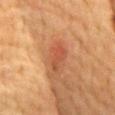Acquisition and patient details: A male subject aged 83 or over. Imaged with cross-polarized lighting. Approximately 3.5 mm at its widest. A lesion tile, about 15 mm wide, cut from a 3D total-body photograph. The lesion is on the front of the torso. The total-body-photography lesion software estimated an average lesion color of about L≈45 a*≈26 b*≈33 (CIELAB), roughly 8 lightness units darker than nearby skin, and a normalized lesion–skin contrast near 6. And it measured a within-lesion color-variation index near 3.5/10 and peripheral color asymmetry of about 1. The analysis additionally found a classifier nevus-likeness of about 35/100 and a detector confidence of about 100 out of 100 that the crop contains a lesion.